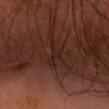Captured during whole-body skin photography for melanoma surveillance; the lesion was not biopsied.